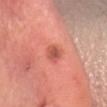Clinical impression: The lesion was photographed on a routine skin check and not biopsied; there is no pathology result. Background: An algorithmic analysis of the crop reported an area of roughly 4 mm², a shape eccentricity near 0.45, and a shape-asymmetry score of about 0.15 (0 = symmetric). This is a white-light tile. The lesion is located on the head or neck. A female subject approximately 40 years of age. Measured at roughly 2 mm in maximum diameter. A roughly 15 mm field-of-view crop from a total-body skin photograph.